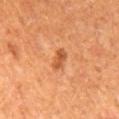A male patient aged around 65.
The total-body-photography lesion software estimated a nevus-likeness score of about 20/100.
Captured under cross-polarized illumination.
Cropped from a total-body skin-imaging series; the visible field is about 15 mm.
Longest diameter approximately 3 mm.
Located on the mid back.